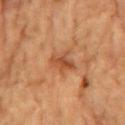| field | value |
|---|---|
| follow-up | catalogued during a skin exam; not biopsied |
| size | about 3 mm |
| tile lighting | cross-polarized illumination |
| image | ~15 mm crop, total-body skin-cancer survey |
| TBP lesion metrics | a lesion area of about 6 mm², an eccentricity of roughly 0.5, and a symmetry-axis asymmetry near 0.25; a border-irregularity rating of about 3.5/10, a within-lesion color-variation index near 3.5/10, and a peripheral color-asymmetry measure near 1.5; an automated nevus-likeness rating near 0 out of 100 and a detector confidence of about 100 out of 100 that the crop contains a lesion |
| body site | the chest |
| patient | male, in their mid- to late 80s or older |